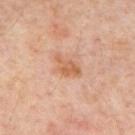Captured during whole-body skin photography for melanoma surveillance; the lesion was not biopsied.
A male patient approximately 65 years of age.
The total-body-photography lesion software estimated an area of roughly 5.5 mm², an eccentricity of roughly 0.8, and a symmetry-axis asymmetry near 0.45. The software also gave a mean CIELAB color near L≈58 a*≈22 b*≈34, roughly 8 lightness units darker than nearby skin, and a normalized border contrast of about 7. The software also gave a border-irregularity rating of about 4.5/10, internal color variation of about 2.5 on a 0–10 scale, and peripheral color asymmetry of about 0.5. And it measured a classifier nevus-likeness of about 10/100 and lesion-presence confidence of about 100/100.
The tile uses cross-polarized illumination.
Approximately 3.5 mm at its widest.
A 15 mm close-up tile from a total-body photography series done for melanoma screening.
The lesion is located on the mid back.